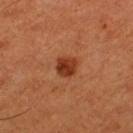notes=imaged on a skin check; not biopsied | body site=the leg | image=total-body-photography crop, ~15 mm field of view | automated metrics=a shape eccentricity near 0.35 and a symmetry-axis asymmetry near 0.2; a classifier nevus-likeness of about 95/100 | patient=male, aged 48 to 52.